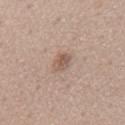From the mid back. About 2.5 mm across. A lesion tile, about 15 mm wide, cut from a 3D total-body photograph. This is a white-light tile. A male subject aged 53–57.